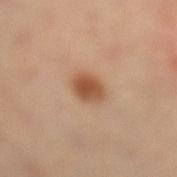Assessment:
No biopsy was performed on this lesion — it was imaged during a full skin examination and was not determined to be concerning.
Acquisition and patient details:
On the right leg. Imaged with cross-polarized lighting. A region of skin cropped from a whole-body photographic capture, roughly 15 mm wide. A female patient aged 38 to 42. Automated tile analysis of the lesion measured a lesion color around L≈52 a*≈22 b*≈34 in CIELAB, roughly 12 lightness units darker than nearby skin, and a normalized border contrast of about 9. The analysis additionally found a border-irregularity rating of about 1.5/10, a color-variation rating of about 3/10, and radial color variation of about 1. It also reported a nevus-likeness score of about 100/100 and a lesion-detection confidence of about 100/100. Approximately 3 mm at its widest.